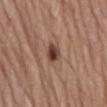notes = no biopsy performed (imaged during a skin exam)
image = 15 mm crop, total-body photography
patient = male, aged approximately 80
body site = the abdomen